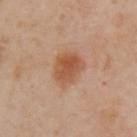  biopsy_status: not biopsied; imaged during a skin examination
  automated_metrics:
    area_mm2_approx: 12.0
    eccentricity: 0.6
    shape_asymmetry: 0.2
    border_irregularity_0_10: 2.0
    color_variation_0_10: 4.5
    peripheral_color_asymmetry: 1.5
    nevus_likeness_0_100: 100
    lesion_detection_confidence_0_100: 100
  lesion_size:
    long_diameter_mm_approx: 4.0
  patient:
    sex: female
    age_approx: 40
  site: left upper arm
  image:
    source: total-body photography crop
    field_of_view_mm: 15